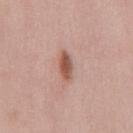<tbp_lesion>
  <biopsy_status>not biopsied; imaged during a skin examination</biopsy_status>
  <lesion_size>
    <long_diameter_mm_approx>3.5</long_diameter_mm_approx>
  </lesion_size>
  <site>chest</site>
  <patient>
    <sex>female</sex>
    <age_approx>40</age_approx>
  </patient>
  <automated_metrics>
    <area_mm2_approx>5.0</area_mm2_approx>
    <eccentricity>0.9</eccentricity>
    <shape_asymmetry>0.25</shape_asymmetry>
    <cielab_L>55</cielab_L>
    <cielab_a>23</cielab_a>
    <cielab_b>27</cielab_b>
    <vs_skin_darker_L>13.0</vs_skin_darker_L>
    <vs_skin_contrast_norm>9.0</vs_skin_contrast_norm>
    <border_irregularity_0_10>2.5</border_irregularity_0_10>
    <color_variation_0_10>4.0</color_variation_0_10>
    <nevus_likeness_0_100>100</nevus_likeness_0_100>
    <lesion_detection_confidence_0_100>100</lesion_detection_confidence_0_100>
  </automated_metrics>
  <image>
    <source>total-body photography crop</source>
    <field_of_view_mm>15</field_of_view_mm>
  </image>
  <lighting>white-light</lighting>
</tbp_lesion>